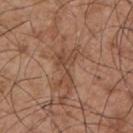biopsy status: catalogued during a skin exam; not biopsied | subject: male, about 55 years old | diameter: ≈5 mm | body site: the chest | acquisition: 15 mm crop, total-body photography | lighting: white-light | automated metrics: a lesion area of about 8.5 mm², an eccentricity of roughly 0.85, and a symmetry-axis asymmetry near 0.7; a lesion–skin lightness drop of about 8 and a lesion-to-skin contrast of about 6 (normalized; higher = more distinct); a nevus-likeness score of about 0/100 and a detector confidence of about 55 out of 100 that the crop contains a lesion.